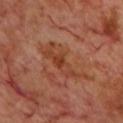Q: Is there a histopathology result?
A: catalogued during a skin exam; not biopsied
Q: What is the imaging modality?
A: ~15 mm tile from a whole-body skin photo
Q: Where on the body is the lesion?
A: the chest
Q: Who is the patient?
A: male, approximately 70 years of age
Q: Illumination type?
A: cross-polarized illumination